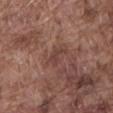Q: Was a biopsy performed?
A: imaged on a skin check; not biopsied
Q: Lesion location?
A: the abdomen
Q: How was this image acquired?
A: 15 mm crop, total-body photography
Q: Automated lesion metrics?
A: a shape eccentricity near 0.85 and a shape-asymmetry score of about 0.5 (0 = symmetric); a detector confidence of about 95 out of 100 that the crop contains a lesion
Q: Illumination type?
A: white-light
Q: How large is the lesion?
A: ≈3 mm
Q: Who is the patient?
A: male, about 75 years old